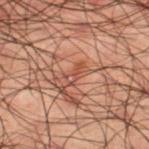Clinical impression:
No biopsy was performed on this lesion — it was imaged during a full skin examination and was not determined to be concerning.
Image and clinical context:
A male subject aged around 50. Automated tile analysis of the lesion measured a shape eccentricity near 0.95 and a symmetry-axis asymmetry near 0.35. About 2.5 mm across. Located on the right thigh. A region of skin cropped from a whole-body photographic capture, roughly 15 mm wide.